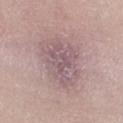biopsy status — imaged on a skin check; not biopsied
tile lighting — white-light
lesion size — ≈6.5 mm
image source — 15 mm crop, total-body photography
subject — female, aged around 25
anatomic site — the right lower leg